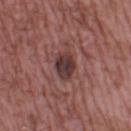Captured during whole-body skin photography for melanoma surveillance; the lesion was not biopsied. Located on the left lower leg. A male patient, about 55 years old. A region of skin cropped from a whole-body photographic capture, roughly 15 mm wide. The recorded lesion diameter is about 3 mm.